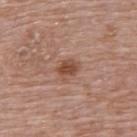No biopsy was performed on this lesion — it was imaged during a full skin examination and was not determined to be concerning.